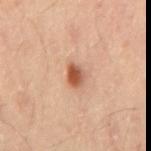follow-up: no biopsy performed (imaged during a skin exam) | subject: male, roughly 55 years of age | location: the back | image: 15 mm crop, total-body photography.